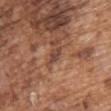The lesion was tiled from a total-body skin photograph and was not biopsied. A roughly 15 mm field-of-view crop from a total-body skin photograph. About 2.5 mm across. The patient is a male aged 73 to 77. The total-body-photography lesion software estimated border irregularity of about 3.5 on a 0–10 scale and peripheral color asymmetry of about 0.5. Imaged with white-light lighting. On the back.